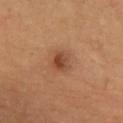biopsy status: total-body-photography surveillance lesion; no biopsy
diameter: ~2.5 mm (longest diameter)
image: total-body-photography crop, ~15 mm field of view
subject: male, aged 53 to 57
tile lighting: cross-polarized illumination
site: the chest